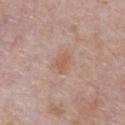| key | value |
|---|---|
| follow-up | total-body-photography surveillance lesion; no biopsy |
| anatomic site | the chest |
| image-analysis metrics | an outline eccentricity of about 0.75 (0 = round, 1 = elongated) and a symmetry-axis asymmetry near 0.3; an average lesion color of about L≈58 a*≈19 b*≈29 (CIELAB), roughly 7 lightness units darker than nearby skin, and a normalized border contrast of about 6 |
| subject | male, about 70 years old |
| lesion size | ≈3 mm |
| image source | 15 mm crop, total-body photography |
| illumination | white-light |